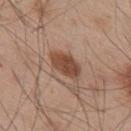Imaged during a routine full-body skin examination; the lesion was not biopsied and no histopathology is available.
Approximately 4 mm at its widest.
The lesion is on the upper back.
This is a white-light tile.
Cropped from a total-body skin-imaging series; the visible field is about 15 mm.
An algorithmic analysis of the crop reported an average lesion color of about L≈46 a*≈21 b*≈29 (CIELAB) and a normalized lesion–skin contrast near 10. And it measured a border-irregularity index near 2/10 and internal color variation of about 3 on a 0–10 scale.
A male subject in their mid-50s.